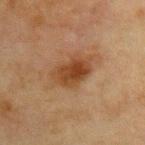Part of a total-body skin-imaging series; this lesion was reviewed on a skin check and was not flagged for biopsy. The lesion is located on the chest. Captured under cross-polarized illumination. The recorded lesion diameter is about 4 mm. Automated image analysis of the tile measured a lesion color around L≈34 a*≈19 b*≈30 in CIELAB, roughly 9 lightness units darker than nearby skin, and a normalized border contrast of about 9. The software also gave a border-irregularity rating of about 2/10, a color-variation rating of about 4.5/10, and peripheral color asymmetry of about 1.5. A male subject approximately 65 years of age. A region of skin cropped from a whole-body photographic capture, roughly 15 mm wide.